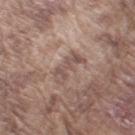subject: male, aged approximately 75 | imaging modality: 15 mm crop, total-body photography | site: the right upper arm | lesion size: ~4 mm (longest diameter).